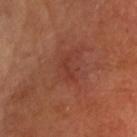Q: Patient demographics?
A: male, about 70 years old
Q: What lighting was used for the tile?
A: cross-polarized illumination
Q: Lesion location?
A: the head or neck
Q: Automated lesion metrics?
A: an average lesion color of about L≈36 a*≈27 b*≈28 (CIELAB), a lesion–skin lightness drop of about 5, and a normalized border contrast of about 5; a border-irregularity index near 6.5/10, internal color variation of about 0 on a 0–10 scale, and peripheral color asymmetry of about 0; lesion-presence confidence of about 100/100
Q: What is the imaging modality?
A: ~15 mm crop, total-body skin-cancer survey